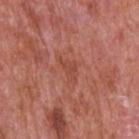The lesion was photographed on a routine skin check and not biopsied; there is no pathology result. This is a white-light tile. A 15 mm close-up tile from a total-body photography series done for melanoma screening. The lesion is on the upper back. The lesion's longest dimension is about 3 mm. The subject is a male about 65 years old.